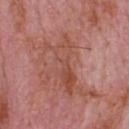Impression:
Captured during whole-body skin photography for melanoma surveillance; the lesion was not biopsied.
Acquisition and patient details:
An algorithmic analysis of the crop reported a footprint of about 12 mm², an eccentricity of roughly 0.95, and two-axis asymmetry of about 0.5. It also reported an average lesion color of about L≈49 a*≈26 b*≈29 (CIELAB), roughly 7 lightness units darker than nearby skin, and a normalized lesion–skin contrast near 6. The lesion is located on the upper back. About 7 mm across. A male patient, aged around 65. This is a white-light tile. A 15 mm close-up tile from a total-body photography series done for melanoma screening.